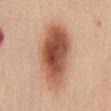  automated_metrics:
    eccentricity: 0.85
    shape_asymmetry: 0.15
    border_irregularity_0_10: 2.0
    color_variation_0_10: 9.5
    peripheral_color_asymmetry: 3.0
  image:
    source: total-body photography crop
    field_of_view_mm: 15
  site: chest
  lighting: white-light
  lesion_size:
    long_diameter_mm_approx: 9.0
  patient:
    sex: female
    age_approx: 50